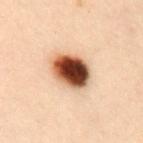follow-up: total-body-photography surveillance lesion; no biopsy | anatomic site: the chest | image: ~15 mm tile from a whole-body skin photo | illumination: cross-polarized | subject: female, roughly 30 years of age | lesion diameter: ~5 mm (longest diameter) | automated lesion analysis: an outline eccentricity of about 0.6 (0 = round, 1 = elongated) and a shape-asymmetry score of about 0.1 (0 = symmetric); a border-irregularity rating of about 1/10 and radial color variation of about 3; a nevus-likeness score of about 100/100 and lesion-presence confidence of about 100/100.